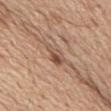<case>
<biopsy_status>not biopsied; imaged during a skin examination</biopsy_status>
<automated_metrics>
  <area_mm2_approx>4.0</area_mm2_approx>
  <eccentricity>0.9</eccentricity>
  <shape_asymmetry>0.35</shape_asymmetry>
  <nevus_likeness_0_100>95</nevus_likeness_0_100>
  <lesion_detection_confidence_0_100>100</lesion_detection_confidence_0_100>
</automated_metrics>
<patient>
  <sex>male</sex>
  <age_approx>70</age_approx>
</patient>
<lesion_size>
  <long_diameter_mm_approx>3.5</long_diameter_mm_approx>
</lesion_size>
<site>mid back</site>
<lighting>white-light</lighting>
<image>
  <source>total-body photography crop</source>
  <field_of_view_mm>15</field_of_view_mm>
</image>
</case>